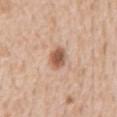The lesion was photographed on a routine skin check and not biopsied; there is no pathology result. On the mid back. Imaged with white-light lighting. A 15 mm crop from a total-body photograph taken for skin-cancer surveillance. A male patient about 60 years old. About 3 mm across.